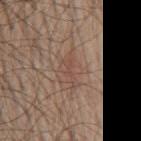follow-up: total-body-photography surveillance lesion; no biopsy | lesion diameter: ≈3.5 mm | image: ~15 mm crop, total-body skin-cancer survey | lighting: white-light illumination | subject: male, approximately 70 years of age | anatomic site: the mid back.